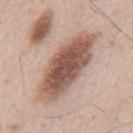| feature | finding |
|---|---|
| notes | total-body-photography surveillance lesion; no biopsy |
| site | the mid back |
| lighting | white-light |
| imaging modality | ~15 mm crop, total-body skin-cancer survey |
| diameter | ≈9.5 mm |
| automated metrics | a shape eccentricity near 0.9 and a symmetry-axis asymmetry near 0.1; an average lesion color of about L≈55 a*≈20 b*≈26 (CIELAB) and a normalized lesion–skin contrast near 10.5; a border-irregularity rating of about 2/10, internal color variation of about 6 on a 0–10 scale, and peripheral color asymmetry of about 1.5 |
| subject | male, aged around 55 |